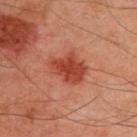notes: total-body-photography surveillance lesion; no biopsy | illumination: cross-polarized | subject: male, aged approximately 50 | image: ~15 mm tile from a whole-body skin photo | automated metrics: roughly 12 lightness units darker than nearby skin and a normalized border contrast of about 9; an automated nevus-likeness rating near 90 out of 100 | size: ~4.5 mm (longest diameter) | location: the back.